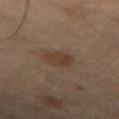Clinical impression:
The lesion was photographed on a routine skin check and not biopsied; there is no pathology result.
Acquisition and patient details:
Measured at roughly 3.5 mm in maximum diameter. A region of skin cropped from a whole-body photographic capture, roughly 15 mm wide. The lesion-visualizer software estimated a border-irregularity index near 2.5/10. Imaged with cross-polarized lighting. The subject is a male aged 68–72. The lesion is on the abdomen.